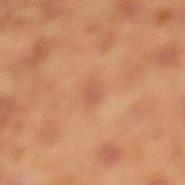Part of a total-body skin-imaging series; this lesion was reviewed on a skin check and was not flagged for biopsy.
A male patient, approximately 45 years of age.
A 15 mm close-up extracted from a 3D total-body photography capture.
Measured at roughly 2.5 mm in maximum diameter.
This is a cross-polarized tile.
On the left lower leg.